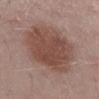patient: male, aged 53 to 57
illumination: white-light illumination
lesion diameter: ~10 mm (longest diameter)
image: ~15 mm tile from a whole-body skin photo
TBP lesion metrics: an area of roughly 49 mm², an outline eccentricity of about 0.7 (0 = round, 1 = elongated), and a shape-asymmetry score of about 0.15 (0 = symmetric); an average lesion color of about L≈49 a*≈19 b*≈23 (CIELAB) and a lesion–skin lightness drop of about 10; a border-irregularity index near 2.5/10, a color-variation rating of about 4/10, and radial color variation of about 1
site: the mid back